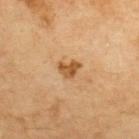Q: Was a biopsy performed?
A: total-body-photography surveillance lesion; no biopsy
Q: What kind of image is this?
A: 15 mm crop, total-body photography
Q: Lesion location?
A: the upper back
Q: Who is the patient?
A: male, about 70 years old
Q: Lesion size?
A: ≈2.5 mm
Q: Illumination type?
A: cross-polarized
Q: Automated lesion metrics?
A: an outline eccentricity of about 0.65 (0 = round, 1 = elongated) and a shape-asymmetry score of about 0.3 (0 = symmetric); a mean CIELAB color near L≈51 a*≈20 b*≈40; a classifier nevus-likeness of about 45/100